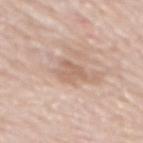The lesion was photographed on a routine skin check and not biopsied; there is no pathology result.
The lesion is on the mid back.
A 15 mm close-up tile from a total-body photography series done for melanoma screening.
This is a white-light tile.
A female patient about 85 years old or older.
Longest diameter approximately 3 mm.
Automated image analysis of the tile measured an automated nevus-likeness rating near 0 out of 100 and a detector confidence of about 100 out of 100 that the crop contains a lesion.